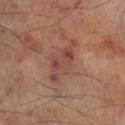Recorded during total-body skin imaging; not selected for excision or biopsy.
A roughly 15 mm field-of-view crop from a total-body skin photograph.
The lesion-visualizer software estimated an eccentricity of roughly 0.9 and a shape-asymmetry score of about 0.3 (0 = symmetric). It also reported a border-irregularity rating of about 5/10, a color-variation rating of about 5.5/10, and peripheral color asymmetry of about 2.
The patient is a male aged approximately 70.
Located on the right lower leg.
Longest diameter approximately 6 mm.